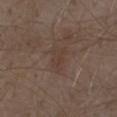This is a white-light tile. Measured at roughly 3 mm in maximum diameter. Cropped from a total-body skin-imaging series; the visible field is about 15 mm. A male patient aged 68 to 72. On the abdomen.